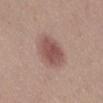Q: Was this lesion biopsied?
A: total-body-photography surveillance lesion; no biopsy
Q: Illumination type?
A: white-light illumination
Q: What did automated image analysis measure?
A: an average lesion color of about L≈51 a*≈21 b*≈23 (CIELAB), about 11 CIELAB-L* units darker than the surrounding skin, and a normalized border contrast of about 8
Q: What kind of image is this?
A: total-body-photography crop, ~15 mm field of view
Q: What is the lesion's diameter?
A: ~4.5 mm (longest diameter)
Q: Lesion location?
A: the leg
Q: Patient demographics?
A: female, aged 18 to 22